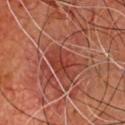This image is a 15 mm lesion crop taken from a total-body photograph.
A male patient, aged 63–67.
The recorded lesion diameter is about 4.5 mm.
Imaged with cross-polarized lighting.
Located on the chest.